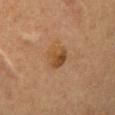* biopsy status: imaged on a skin check; not biopsied
* patient: male, approximately 60 years of age
* illumination: cross-polarized illumination
* image source: 15 mm crop, total-body photography
* anatomic site: the front of the torso
* image-analysis metrics: an area of roughly 6.5 mm² and a symmetry-axis asymmetry near 0.2; a nevus-likeness score of about 45/100 and a detector confidence of about 100 out of 100 that the crop contains a lesion